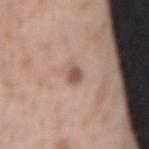biopsy_status: not biopsied; imaged during a skin examination
image:
  source: total-body photography crop
  field_of_view_mm: 15
automated_metrics:
  area_mm2_approx: 3.0
  eccentricity: 0.75
  shape_asymmetry: 0.2
  cielab_L: 53
  cielab_a: 20
  cielab_b: 25
  vs_skin_darker_L: 12.0
  vs_skin_contrast_norm: 8.0
  nevus_likeness_0_100: 95
  lesion_detection_confidence_0_100: 100
patient:
  sex: male
  age_approx: 45
lesion_size:
  long_diameter_mm_approx: 2.5
site: lower back